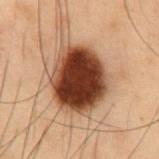workup=imaged on a skin check; not biopsied
location=the abdomen
imaging modality=~15 mm crop, total-body skin-cancer survey
lesion diameter=~7 mm (longest diameter)
illumination=cross-polarized illumination
patient=male, aged 53–57